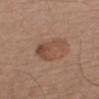Recorded during total-body skin imaging; not selected for excision or biopsy.
This image is a 15 mm lesion crop taken from a total-body photograph.
Automated tile analysis of the lesion measured a mean CIELAB color near L≈48 a*≈20 b*≈28 and a lesion–skin lightness drop of about 8. The analysis additionally found border irregularity of about 6.5 on a 0–10 scale and a within-lesion color-variation index near 4.5/10. The software also gave a nevus-likeness score of about 35/100 and lesion-presence confidence of about 100/100.
Longest diameter approximately 4.5 mm.
The patient is a male aged around 75.
The tile uses white-light illumination.
The lesion is located on the back.